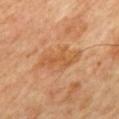Part of a total-body skin-imaging series; this lesion was reviewed on a skin check and was not flagged for biopsy. A patient in their mid-60s. Located on the mid back. Automated image analysis of the tile measured a shape eccentricity near 0.95. And it measured a classifier nevus-likeness of about 0/100 and lesion-presence confidence of about 100/100. The recorded lesion diameter is about 5.5 mm. A 15 mm close-up tile from a total-body photography series done for melanoma screening. The tile uses cross-polarized illumination.